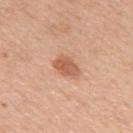This lesion was catalogued during total-body skin photography and was not selected for biopsy. This is a white-light tile. About 3.5 mm across. On the upper back. A 15 mm close-up extracted from a 3D total-body photography capture. A female patient, aged 43–47. Automated tile analysis of the lesion measured border irregularity of about 2 on a 0–10 scale and peripheral color asymmetry of about 1.